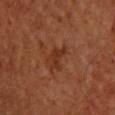Assessment:
Captured during whole-body skin photography for melanoma surveillance; the lesion was not biopsied.
Context:
The lesion is on the upper back. A male patient, roughly 60 years of age. An algorithmic analysis of the crop reported an area of roughly 4 mm², an outline eccentricity of about 0.85 (0 = round, 1 = elongated), and a shape-asymmetry score of about 0.5 (0 = symmetric). The software also gave an average lesion color of about L≈32 a*≈25 b*≈31 (CIELAB), a lesion–skin lightness drop of about 7, and a normalized lesion–skin contrast near 7. The analysis additionally found an automated nevus-likeness rating near 0 out of 100 and a lesion-detection confidence of about 100/100. The tile uses cross-polarized illumination. A 15 mm crop from a total-body photograph taken for skin-cancer surveillance.